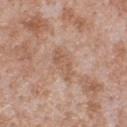  biopsy_status: not biopsied; imaged during a skin examination
  lighting: white-light
  patient:
    sex: male
    age_approx: 65
  site: chest
  image:
    source: total-body photography crop
    field_of_view_mm: 15
  lesion_size:
    long_diameter_mm_approx: 4.5